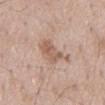Recorded during total-body skin imaging; not selected for excision or biopsy. A 15 mm close-up tile from a total-body photography series done for melanoma screening. A male patient aged 58–62. Automated image analysis of the tile measured an area of roughly 9 mm² and a symmetry-axis asymmetry near 0.4. And it measured a border-irregularity rating of about 5/10, a color-variation rating of about 3/10, and radial color variation of about 1. The lesion is located on the mid back. The tile uses white-light illumination. About 4.5 mm across.